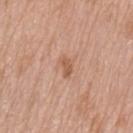Impression: Captured during whole-body skin photography for melanoma surveillance; the lesion was not biopsied. Image and clinical context: The lesion-visualizer software estimated a lesion area of about 3.5 mm², a shape eccentricity near 0.8, and two-axis asymmetry of about 0.35. And it measured a lesion color around L≈58 a*≈21 b*≈32 in CIELAB, a lesion–skin lightness drop of about 8, and a normalized border contrast of about 6. On the back. A close-up tile cropped from a whole-body skin photograph, about 15 mm across. The lesion's longest dimension is about 2.5 mm. The subject is a female about 75 years old.